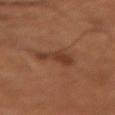| feature | finding |
|---|---|
| biopsy status | no biopsy performed (imaged during a skin exam) |
| acquisition | 15 mm crop, total-body photography |
| image-analysis metrics | an area of roughly 5 mm², an outline eccentricity of about 0.85 (0 = round, 1 = elongated), and a shape-asymmetry score of about 0.6 (0 = symmetric); an average lesion color of about L≈37 a*≈23 b*≈30 (CIELAB), roughly 8 lightness units darker than nearby skin, and a normalized lesion–skin contrast near 7; an automated nevus-likeness rating near 15 out of 100 |
| site | the right upper arm |
| patient | male, aged 48 to 52 |
| lighting | cross-polarized illumination |